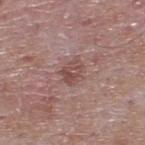Image and clinical context: This is a white-light tile. The patient is a male aged around 65. A lesion tile, about 15 mm wide, cut from a 3D total-body photograph. From the upper back.